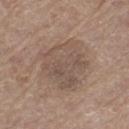notes: catalogued during a skin exam; not biopsied | acquisition: 15 mm crop, total-body photography | subject: male, about 65 years old | body site: the left thigh.